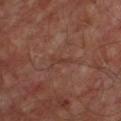biopsy status — catalogued during a skin exam; not biopsied | patient — male, in their mid- to late 50s | automated lesion analysis — a lesion area of about 2 mm² and a shape eccentricity near 0.95; a border-irregularity index near 4/10 and a peripheral color-asymmetry measure near 0 | anatomic site — the chest | image source — total-body-photography crop, ~15 mm field of view | illumination — cross-polarized | size — ~3 mm (longest diameter).